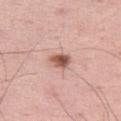  biopsy_status: not biopsied; imaged during a skin examination
  automated_metrics:
    cielab_L: 56
    cielab_a: 23
    cielab_b: 26
    vs_skin_darker_L: 16.0
    vs_skin_contrast_norm: 10.0
    border_irregularity_0_10: 2.5
    color_variation_0_10: 6.5
    peripheral_color_asymmetry: 2.0
    nevus_likeness_0_100: 95
  site: left thigh
  lighting: white-light
  image:
    source: total-body photography crop
    field_of_view_mm: 15
  patient:
    sex: male
    age_approx: 50